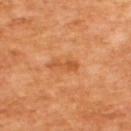notes: no biopsy performed (imaged during a skin exam)
size: ~3 mm (longest diameter)
image-analysis metrics: a shape eccentricity near 0.9 and two-axis asymmetry of about 0.35; a border-irregularity rating of about 4/10, internal color variation of about 1 on a 0–10 scale, and radial color variation of about 0; a classifier nevus-likeness of about 25/100
illumination: cross-polarized
patient: male, aged around 65
acquisition: ~15 mm crop, total-body skin-cancer survey